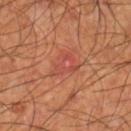  biopsy_status: not biopsied; imaged during a skin examination
  lesion_size:
    long_diameter_mm_approx: 3.5
  lighting: cross-polarized
  image:
    source: total-body photography crop
    field_of_view_mm: 15
  patient:
    sex: male
    age_approx: 60
  automated_metrics:
    eccentricity: 0.7
    shape_asymmetry: 0.55
    cielab_L: 48
    cielab_a: 31
    cielab_b: 31
    vs_skin_darker_L: 6.0
    vs_skin_contrast_norm: 5.0
    nevus_likeness_0_100: 0
    lesion_detection_confidence_0_100: 100
  site: right lower leg